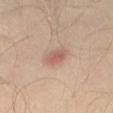Q: Was a biopsy performed?
A: total-body-photography surveillance lesion; no biopsy